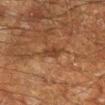follow-up: imaged on a skin check; not biopsied | size: ~2.5 mm (longest diameter) | patient: male, aged approximately 60 | site: the right lower leg | image source: total-body-photography crop, ~15 mm field of view | illumination: cross-polarized.